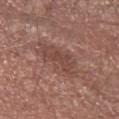This lesion was catalogued during total-body skin photography and was not selected for biopsy.
Cropped from a whole-body photographic skin survey; the tile spans about 15 mm.
The tile uses white-light illumination.
A male subject in their 70s.
About 6 mm across.
Located on the left forearm.